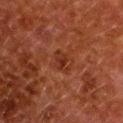The lesion was photographed on a routine skin check and not biopsied; there is no pathology result. Longest diameter approximately 2.5 mm. The lesion is located on the upper back. A female patient approximately 50 years of age. Captured under cross-polarized illumination. A close-up tile cropped from a whole-body skin photograph, about 15 mm across.